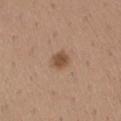workup — catalogued during a skin exam; not biopsied
illumination — white-light illumination
automated metrics — a lesion area of about 4 mm², a shape eccentricity near 0.6, and a symmetry-axis asymmetry near 0.15; a within-lesion color-variation index near 1.5/10 and peripheral color asymmetry of about 0.5; an automated nevus-likeness rating near 90 out of 100 and a detector confidence of about 100 out of 100 that the crop contains a lesion
anatomic site — the left upper arm
patient — male, aged 38 to 42
size — ~2.5 mm (longest diameter)
image source — ~15 mm tile from a whole-body skin photo